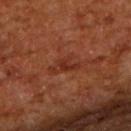Imaged during a routine full-body skin examination; the lesion was not biopsied and no histopathology is available. A 15 mm crop from a total-body photograph taken for skin-cancer surveillance. An algorithmic analysis of the crop reported a footprint of about 5 mm² and an outline eccentricity of about 0.9 (0 = round, 1 = elongated). The analysis additionally found a border-irregularity rating of about 6/10, a color-variation rating of about 2/10, and peripheral color asymmetry of about 0.5. On the upper back. A male subject, aged around 65. About 4 mm across.